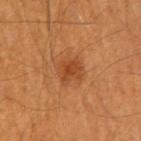workup = catalogued during a skin exam; not biopsied | size = ≈3 mm | anatomic site = the right upper arm | automated lesion analysis = a mean CIELAB color near L≈42 a*≈25 b*≈37, about 8 CIELAB-L* units darker than the surrounding skin, and a normalized border contrast of about 6.5; a border-irregularity index near 2/10 and peripheral color asymmetry of about 1; a classifier nevus-likeness of about 65/100 and a lesion-detection confidence of about 100/100 | acquisition = 15 mm crop, total-body photography | subject = male, approximately 60 years of age.